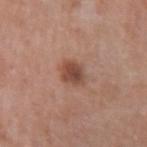<tbp_lesion>
  <biopsy_status>not biopsied; imaged during a skin examination</biopsy_status>
  <site>arm</site>
  <patient>
    <sex>female</sex>
    <age_approx>60</age_approx>
  </patient>
  <automated_metrics>
    <area_mm2_approx>6.0</area_mm2_approx>
    <eccentricity>0.55</eccentricity>
    <shape_asymmetry>0.2</shape_asymmetry>
    <nevus_likeness_0_100>85</nevus_likeness_0_100>
    <lesion_detection_confidence_0_100>100</lesion_detection_confidence_0_100>
  </automated_metrics>
  <image>
    <source>total-body photography crop</source>
    <field_of_view_mm>15</field_of_view_mm>
  </image>
  <lesion_size>
    <long_diameter_mm_approx>3.0</long_diameter_mm_approx>
  </lesion_size>
  <lighting>white-light</lighting>
</tbp_lesion>